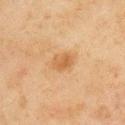Imaged during a routine full-body skin examination; the lesion was not biopsied and no histopathology is available.
About 2.5 mm across.
The lesion is located on the left upper arm.
A male patient, aged 43 to 47.
This image is a 15 mm lesion crop taken from a total-body photograph.